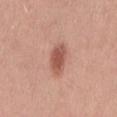follow-up=catalogued during a skin exam; not biopsied
imaging modality=~15 mm crop, total-body skin-cancer survey
patient=male, aged 28–32
site=the back